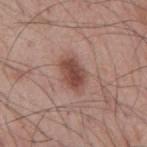| field | value |
|---|---|
| follow-up | total-body-photography surveillance lesion; no biopsy |
| acquisition | ~15 mm tile from a whole-body skin photo |
| site | the mid back |
| tile lighting | white-light |
| patient | male, aged 53–57 |
| size | ≈4.5 mm |